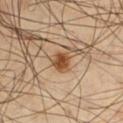Part of a total-body skin-imaging series; this lesion was reviewed on a skin check and was not flagged for biopsy.